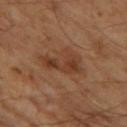follow-up: total-body-photography surveillance lesion; no biopsy
subject: male, about 65 years old
illumination: cross-polarized illumination
lesion size: ~5 mm (longest diameter)
automated metrics: a footprint of about 9 mm² and an outline eccentricity of about 0.85 (0 = round, 1 = elongated)
image: total-body-photography crop, ~15 mm field of view